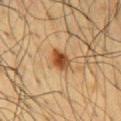Captured during whole-body skin photography for melanoma surveillance; the lesion was not biopsied. Measured at roughly 3 mm in maximum diameter. The patient is a male aged 58 to 62. The total-body-photography lesion software estimated internal color variation of about 6 on a 0–10 scale and peripheral color asymmetry of about 2. And it measured an automated nevus-likeness rating near 95 out of 100 and lesion-presence confidence of about 100/100. The lesion is on the chest. A close-up tile cropped from a whole-body skin photograph, about 15 mm across. Captured under cross-polarized illumination.